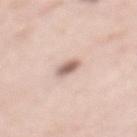biopsy_status: not biopsied; imaged during a skin examination
image:
  source: total-body photography crop
  field_of_view_mm: 15
site: back
lesion_size:
  long_diameter_mm_approx: 2.5
patient:
  sex: female
  age_approx: 40